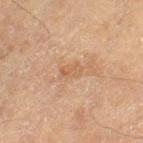Longest diameter approximately 2.5 mm.
A lesion tile, about 15 mm wide, cut from a 3D total-body photograph.
The patient is a male about 70 years old.
On the left thigh.
Imaged with cross-polarized lighting.